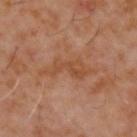{"site": "back", "image": {"source": "total-body photography crop", "field_of_view_mm": 15}, "patient": {"sex": "male", "age_approx": 60}, "lighting": "cross-polarized", "lesion_size": {"long_diameter_mm_approx": 6.0}, "automated_metrics": {"vs_skin_darker_L": 6.0, "vs_skin_contrast_norm": 5.5, "border_irregularity_0_10": 6.5, "color_variation_0_10": 2.5}}